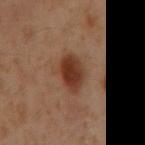No biopsy was performed on this lesion — it was imaged during a full skin examination and was not determined to be concerning. A male patient, aged around 60. A 15 mm close-up extracted from a 3D total-body photography capture. From the mid back. This is a cross-polarized tile. The lesion's longest dimension is about 4.5 mm.